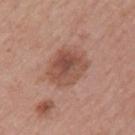Notes:
• workup · catalogued during a skin exam; not biopsied
• patient · female, in their mid-50s
• lighting · white-light illumination
• lesion size · about 5 mm
• automated lesion analysis · an average lesion color of about L≈50 a*≈22 b*≈28 (CIELAB) and a normalized border contrast of about 7.5; an automated nevus-likeness rating near 45 out of 100 and a detector confidence of about 100 out of 100 that the crop contains a lesion
• site · the left upper arm
• acquisition · ~15 mm tile from a whole-body skin photo